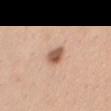  biopsy_status: not biopsied; imaged during a skin examination
  site: chest
  lighting: white-light
  patient:
    sex: female
    age_approx: 30
  lesion_size:
    long_diameter_mm_approx: 2.5
  image:
    source: total-body photography crop
    field_of_view_mm: 15
  automated_metrics:
    area_mm2_approx: 4.5
    eccentricity: 0.75
    shape_asymmetry: 0.25
    border_irregularity_0_10: 2.0
    color_variation_0_10: 3.5
    peripheral_color_asymmetry: 1.0